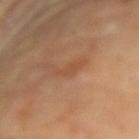Q: Is there a histopathology result?
A: catalogued during a skin exam; not biopsied